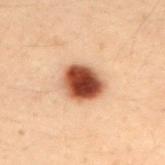Captured during whole-body skin photography for melanoma surveillance; the lesion was not biopsied.